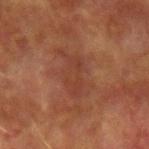No biopsy was performed on this lesion — it was imaged during a full skin examination and was not determined to be concerning.
On the arm.
A male patient, aged 73–77.
A 15 mm crop from a total-body photograph taken for skin-cancer surveillance.
Longest diameter approximately 5.5 mm.